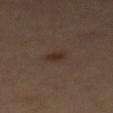follow-up: imaged on a skin check; not biopsied
patient: male, aged 58–62
lesion diameter: ≈2.5 mm
tile lighting: cross-polarized
image-analysis metrics: a lesion area of about 3 mm², an outline eccentricity of about 0.8 (0 = round, 1 = elongated), and a symmetry-axis asymmetry near 0.15; roughly 7 lightness units darker than nearby skin and a normalized border contrast of about 7.5; a border-irregularity rating of about 1.5/10, internal color variation of about 1 on a 0–10 scale, and peripheral color asymmetry of about 0.5; a lesion-detection confidence of about 100/100
location: the mid back
imaging modality: total-body-photography crop, ~15 mm field of view